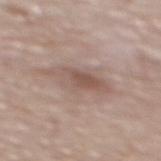Part of a total-body skin-imaging series; this lesion was reviewed on a skin check and was not flagged for biopsy. A roughly 15 mm field-of-view crop from a total-body skin photograph. The tile uses white-light illumination. A male patient, aged around 85 or older. The lesion is located on the upper back.